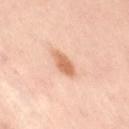Assessment: Imaged during a routine full-body skin examination; the lesion was not biopsied and no histopathology is available. Clinical summary: Located on the leg. A 15 mm close-up tile from a total-body photography series done for melanoma screening. Imaged with cross-polarized lighting. An algorithmic analysis of the crop reported an average lesion color of about L≈68 a*≈25 b*≈36 (CIELAB), a lesion–skin lightness drop of about 13, and a lesion-to-skin contrast of about 8 (normalized; higher = more distinct). The subject is a female aged approximately 40.